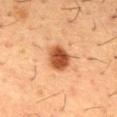Case summary:
• follow-up: imaged on a skin check; not biopsied
• illumination: cross-polarized
• acquisition: 15 mm crop, total-body photography
• patient: male, roughly 55 years of age
• anatomic site: the chest
• automated lesion analysis: an average lesion color of about L≈46 a*≈26 b*≈36 (CIELAB), a lesion–skin lightness drop of about 16, and a normalized border contrast of about 11.5